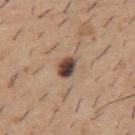Case summary:
- biopsy status — no biopsy performed (imaged during a skin exam)
- patient — male, roughly 40 years of age
- anatomic site — the mid back
- acquisition — ~15 mm crop, total-body skin-cancer survey